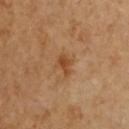follow-up = catalogued during a skin exam; not biopsied | acquisition = ~15 mm tile from a whole-body skin photo | site = the chest | patient = female, in their mid- to late 50s.